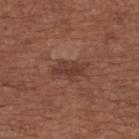| key | value |
|---|---|
| biopsy status | total-body-photography surveillance lesion; no biopsy |
| lighting | white-light illumination |
| subject | female, aged 63–67 |
| lesion diameter | about 4 mm |
| automated metrics | an outline eccentricity of about 0.85 (0 = round, 1 = elongated) and two-axis asymmetry of about 0.35; a mean CIELAB color near L≈38 a*≈21 b*≈25 and a lesion-to-skin contrast of about 7 (normalized; higher = more distinct); a classifier nevus-likeness of about 25/100 and lesion-presence confidence of about 100/100 |
| anatomic site | the upper back |
| acquisition | 15 mm crop, total-body photography |